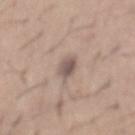The lesion was photographed on a routine skin check and not biopsied; there is no pathology result. Imaged with white-light lighting. The lesion is on the mid back. A male patient, aged around 65. Cropped from a total-body skin-imaging series; the visible field is about 15 mm. Longest diameter approximately 3.5 mm.